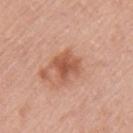<tbp_lesion>
  <biopsy_status>not biopsied; imaged during a skin examination</biopsy_status>
  <lesion_size>
    <long_diameter_mm_approx>3.5</long_diameter_mm_approx>
  </lesion_size>
  <automated_metrics>
    <nevus_likeness_0_100>35</nevus_likeness_0_100>
    <lesion_detection_confidence_0_100>100</lesion_detection_confidence_0_100>
  </automated_metrics>
  <image>
    <source>total-body photography crop</source>
    <field_of_view_mm>15</field_of_view_mm>
  </image>
  <site>left upper arm</site>
  <patient>
    <sex>female</sex>
    <age_approx>40</age_approx>
  </patient>
</tbp_lesion>